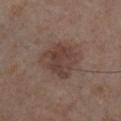Case summary:
– workup — total-body-photography surveillance lesion; no biopsy
– patient — male, roughly 55 years of age
– location — the chest
– imaging modality — ~15 mm crop, total-body skin-cancer survey